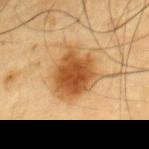Assessment: Imaged during a routine full-body skin examination; the lesion was not biopsied and no histopathology is available. Background: This is a cross-polarized tile. An algorithmic analysis of the crop reported a footprint of about 20 mm² and an eccentricity of roughly 0.35. It also reported a lesion color around L≈47 a*≈21 b*≈38 in CIELAB and a lesion-to-skin contrast of about 10.5 (normalized; higher = more distinct). And it measured border irregularity of about 3 on a 0–10 scale, a color-variation rating of about 4.5/10, and radial color variation of about 1. Longest diameter approximately 5.5 mm. A lesion tile, about 15 mm wide, cut from a 3D total-body photograph. Located on the chest. The subject is a male aged 83 or older.